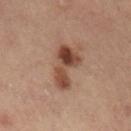No biopsy was performed on this lesion — it was imaged during a full skin examination and was not determined to be concerning.
Measured at roughly 5.5 mm in maximum diameter.
A male patient, aged around 55.
The tile uses cross-polarized illumination.
On the right lower leg.
A 15 mm close-up extracted from a 3D total-body photography capture.
Automated image analysis of the tile measured an area of roughly 11 mm², an eccentricity of roughly 0.85, and a shape-asymmetry score of about 0.4 (0 = symmetric). It also reported an average lesion color of about L≈36 a*≈17 b*≈23 (CIELAB).